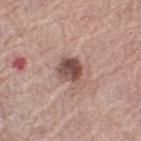Findings:
– notes · no biopsy performed (imaged during a skin exam)
– image · 15 mm crop, total-body photography
– diameter · ≈3 mm
– subject · female, approximately 65 years of age
– anatomic site · the left thigh